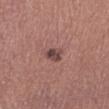The tile uses white-light illumination. A male subject, about 45 years old. The lesion's longest dimension is about 2.5 mm. On the left lower leg. A 15 mm close-up tile from a total-body photography series done for melanoma screening.